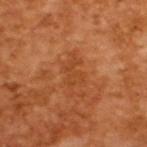Imaged during a routine full-body skin examination; the lesion was not biopsied and no histopathology is available.